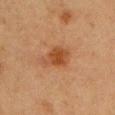  biopsy_status: not biopsied; imaged during a skin examination
  patient:
    sex: female
    age_approx: 40
  image:
    source: total-body photography crop
    field_of_view_mm: 15
  site: left upper arm
  lighting: cross-polarized